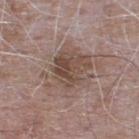{"biopsy_status": "not biopsied; imaged during a skin examination", "image": {"source": "total-body photography crop", "field_of_view_mm": 15}, "automated_metrics": {"area_mm2_approx": 20.0, "eccentricity": 0.55, "cielab_L": 48, "cielab_a": 15, "cielab_b": 23, "vs_skin_darker_L": 9.0, "vs_skin_contrast_norm": 7.5, "border_irregularity_0_10": 5.0, "peripheral_color_asymmetry": 2.0, "lesion_detection_confidence_0_100": 100}, "patient": {"sex": "male", "age_approx": 65}, "site": "upper back", "lighting": "white-light", "lesion_size": {"long_diameter_mm_approx": 6.0}}